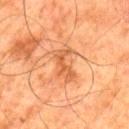follow-up = no biopsy performed (imaged during a skin exam) | patient = male, approximately 80 years of age | lesion size = ≈4.5 mm | imaging modality = ~15 mm tile from a whole-body skin photo | lighting = cross-polarized | location = the left thigh.